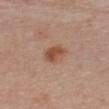Background:
The patient is a female aged approximately 50. Located on the chest. The lesion-visualizer software estimated an eccentricity of roughly 0.65. The analysis additionally found an average lesion color of about L≈51 a*≈21 b*≈30 (CIELAB). It also reported a border-irregularity index near 1.5/10 and a within-lesion color-variation index near 4.5/10. This is a white-light tile. The recorded lesion diameter is about 3 mm. Cropped from a whole-body photographic skin survey; the tile spans about 15 mm.